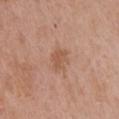Notes:
• workup · no biopsy performed (imaged during a skin exam)
• subject · female, roughly 65 years of age
• image source · ~15 mm crop, total-body skin-cancer survey
• illumination · white-light illumination
• TBP lesion metrics · a mean CIELAB color near L≈55 a*≈22 b*≈32, a lesion–skin lightness drop of about 7, and a lesion-to-skin contrast of about 6 (normalized; higher = more distinct); border irregularity of about 4 on a 0–10 scale, a color-variation rating of about 1.5/10, and a peripheral color-asymmetry measure near 0.5
• site · the chest
• diameter · about 2.5 mm